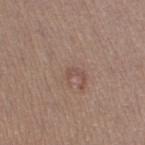The subject is a female about 40 years old. On the leg. A close-up tile cropped from a whole-body skin photograph, about 15 mm across. An algorithmic analysis of the crop reported a lesion color around L≈49 a*≈18 b*≈24 in CIELAB, roughly 6 lightness units darker than nearby skin, and a lesion-to-skin contrast of about 4.5 (normalized; higher = more distinct). The software also gave a nevus-likeness score of about 0/100 and a lesion-detection confidence of about 100/100.